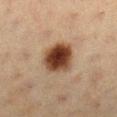<lesion>
<biopsy_status>not biopsied; imaged during a skin examination</biopsy_status>
<lighting>cross-polarized</lighting>
<automated_metrics>
  <area_mm2_approx>13.0</area_mm2_approx>
  <eccentricity>0.25</eccentricity>
  <shape_asymmetry>0.1</shape_asymmetry>
  <cielab_L>37</cielab_L>
  <cielab_a>18</cielab_a>
  <cielab_b>27</cielab_b>
  <vs_skin_darker_L>17.0</vs_skin_darker_L>
  <border_irregularity_0_10>1.0</border_irregularity_0_10>
  <nevus_likeness_0_100>100</nevus_likeness_0_100>
  <lesion_detection_confidence_0_100>100</lesion_detection_confidence_0_100>
</automated_metrics>
<patient>
  <sex>female</sex>
  <age_approx>40</age_approx>
</patient>
<site>leg</site>
<lesion_size>
  <long_diameter_mm_approx>4.0</long_diameter_mm_approx>
</lesion_size>
<image>
  <source>total-body photography crop</source>
  <field_of_view_mm>15</field_of_view_mm>
</image>
</lesion>